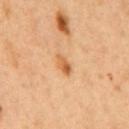Assessment:
This lesion was catalogued during total-body skin photography and was not selected for biopsy.
Clinical summary:
An algorithmic analysis of the crop reported an average lesion color of about L≈61 a*≈24 b*≈42 (CIELAB), about 11 CIELAB-L* units darker than the surrounding skin, and a lesion-to-skin contrast of about 7.5 (normalized; higher = more distinct). Imaged with cross-polarized lighting. From the mid back. A male patient, aged 48–52. Approximately 3 mm at its widest. A region of skin cropped from a whole-body photographic capture, roughly 15 mm wide.